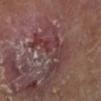location — the leg
diameter — ~6 mm (longest diameter)
image source — ~15 mm tile from a whole-body skin photo
patient — male, aged around 70
illumination — cross-polarized illumination